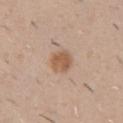The lesion was photographed on a routine skin check and not biopsied; there is no pathology result.
The patient is a male aged approximately 50.
The tile uses white-light illumination.
Automated tile analysis of the lesion measured a nevus-likeness score of about 95/100.
From the chest.
Approximately 3 mm at its widest.
Cropped from a total-body skin-imaging series; the visible field is about 15 mm.